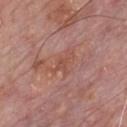Clinical impression: Recorded during total-body skin imaging; not selected for excision or biopsy.